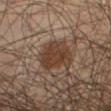| key | value |
|---|---|
| workup | no biopsy performed (imaged during a skin exam) |
| subject | male, approximately 55 years of age |
| body site | the left thigh |
| acquisition | ~15 mm tile from a whole-body skin photo |